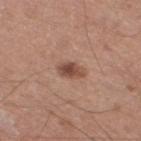workup: total-body-photography surveillance lesion; no biopsy | site: the left lower leg | subject: male, aged around 50 | acquisition: ~15 mm tile from a whole-body skin photo | diameter: ≈3 mm | tile lighting: white-light illumination.